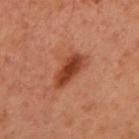{
  "biopsy_status": "not biopsied; imaged during a skin examination",
  "patient": {
    "sex": "female",
    "age_approx": 55
  },
  "image": {
    "source": "total-body photography crop",
    "field_of_view_mm": 15
  },
  "lighting": "cross-polarized",
  "lesion_size": {
    "long_diameter_mm_approx": 4.5
  },
  "automated_metrics": {
    "eccentricity": 0.9,
    "shape_asymmetry": 0.2,
    "color_variation_0_10": 3.5,
    "peripheral_color_asymmetry": 1.0,
    "nevus_likeness_0_100": 95,
    "lesion_detection_confidence_0_100": 100
  },
  "site": "front of the torso"
}